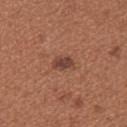<record>
<biopsy_status>not biopsied; imaged during a skin examination</biopsy_status>
<patient>
  <sex>female</sex>
  <age_approx>30</age_approx>
</patient>
<lighting>white-light</lighting>
<lesion_size>
  <long_diameter_mm_approx>2.5</long_diameter_mm_approx>
</lesion_size>
<automated_metrics>
  <area_mm2_approx>4.0</area_mm2_approx>
  <cielab_L>42</cielab_L>
  <cielab_a>22</cielab_a>
  <cielab_b>26</cielab_b>
  <vs_skin_darker_L>10.0</vs_skin_darker_L>
  <vs_skin_contrast_norm>9.0</vs_skin_contrast_norm>
  <border_irregularity_0_10>2.5</border_irregularity_0_10>
  <peripheral_color_asymmetry>1.0</peripheral_color_asymmetry>
  <nevus_likeness_0_100>90</nevus_likeness_0_100>
  <lesion_detection_confidence_0_100>100</lesion_detection_confidence_0_100>
</automated_metrics>
<image>
  <source>total-body photography crop</source>
  <field_of_view_mm>15</field_of_view_mm>
</image>
<site>left upper arm</site>
</record>